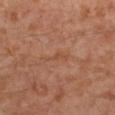biopsy status: total-body-photography surveillance lesion; no biopsy | image source: ~15 mm tile from a whole-body skin photo | tile lighting: cross-polarized illumination | location: the left leg | lesion diameter: ≈3.5 mm | subject: male, aged 28–32.